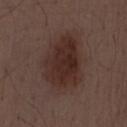| field | value |
|---|---|
| workup | no biopsy performed (imaged during a skin exam) |
| lighting | white-light |
| patient | male, about 55 years old |
| image | total-body-photography crop, ~15 mm field of view |
| size | ≈6.5 mm |
| anatomic site | the mid back |
| TBP lesion metrics | a border-irregularity rating of about 2.5/10, a color-variation rating of about 3.5/10, and radial color variation of about 1.5 |